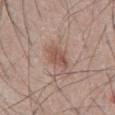This lesion was catalogued during total-body skin photography and was not selected for biopsy.
The total-body-photography lesion software estimated a lesion area of about 5.5 mm² and an outline eccentricity of about 0.8 (0 = round, 1 = elongated).
From the abdomen.
Cropped from a whole-body photographic skin survey; the tile spans about 15 mm.
Imaged with white-light lighting.
Approximately 3.5 mm at its widest.
The patient is a male aged 63–67.